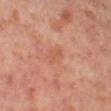Q: Was a biopsy performed?
A: catalogued during a skin exam; not biopsied
Q: What did automated image analysis measure?
A: a lesion color around L≈54 a*≈25 b*≈33 in CIELAB, roughly 6 lightness units darker than nearby skin, and a normalized border contrast of about 4.5; a border-irregularity index near 3.5/10 and a color-variation rating of about 0.5/10
Q: Lesion location?
A: the left thigh
Q: What is the imaging modality?
A: total-body-photography crop, ~15 mm field of view
Q: How was the tile lit?
A: cross-polarized
Q: How large is the lesion?
A: about 3 mm
Q: Who is the patient?
A: female, aged around 50